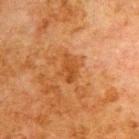Background:
A 15 mm close-up tile from a total-body photography series done for melanoma screening. The patient is a male aged 78 to 82. On the back.